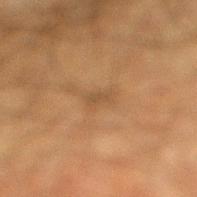tile lighting=cross-polarized
patient=male, aged 48 to 52
acquisition=~15 mm crop, total-body skin-cancer survey
size=~2.5 mm (longest diameter)
anatomic site=the right lower leg